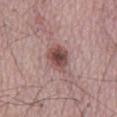Part of a total-body skin-imaging series; this lesion was reviewed on a skin check and was not flagged for biopsy. A region of skin cropped from a whole-body photographic capture, roughly 15 mm wide. Imaged with white-light lighting. A male patient aged approximately 65. From the back.